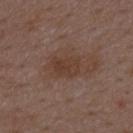Assessment: The lesion was photographed on a routine skin check and not biopsied; there is no pathology result. Background: Approximately 3.5 mm at its widest. Cropped from a total-body skin-imaging series; the visible field is about 15 mm. A male patient aged approximately 50. The lesion is on the mid back. Imaged with white-light lighting.